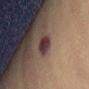workup = imaged on a skin check; not biopsied | size = ~3 mm (longest diameter) | image = 15 mm crop, total-body photography | body site = the abdomen | patient = female, aged 53–57 | tile lighting = cross-polarized | automated lesion analysis = a color-variation rating of about 5/10 and radial color variation of about 2; an automated nevus-likeness rating near 15 out of 100 and a lesion-detection confidence of about 100/100.